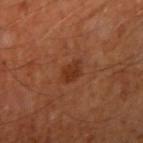image source — ~15 mm tile from a whole-body skin photo
patient — male, aged 58–62
lesion diameter — ~2.5 mm (longest diameter)
automated metrics — a border-irregularity rating of about 2/10, a color-variation rating of about 2/10, and peripheral color asymmetry of about 0.5; a detector confidence of about 100 out of 100 that the crop contains a lesion
site — the arm